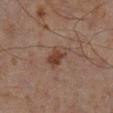biopsy status: total-body-photography surveillance lesion; no biopsy | image source: total-body-photography crop, ~15 mm field of view | location: the left lower leg | diameter: about 3 mm | patient: male, aged approximately 65.